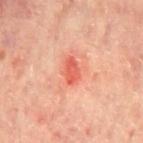<record>
<biopsy_status>not biopsied; imaged during a skin examination</biopsy_status>
<image>
  <source>total-body photography crop</source>
  <field_of_view_mm>15</field_of_view_mm>
</image>
<patient>
  <sex>male</sex>
  <age_approx>65</age_approx>
</patient>
<lighting>cross-polarized</lighting>
<automated_metrics>
  <eccentricity>0.75</eccentricity>
  <shape_asymmetry>0.2</shape_asymmetry>
  <cielab_L>61</cielab_L>
  <cielab_a>38</cielab_a>
  <cielab_b>35</cielab_b>
  <vs_skin_contrast_norm>7.0</vs_skin_contrast_norm>
  <border_irregularity_0_10>2.0</border_irregularity_0_10>
  <color_variation_0_10>3.0</color_variation_0_10>
  <peripheral_color_asymmetry>1.0</peripheral_color_asymmetry>
</automated_metrics>
<site>mid back</site>
<lesion_size>
  <long_diameter_mm_approx>3.0</long_diameter_mm_approx>
</lesion_size>
</record>